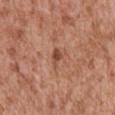Q: Is there a histopathology result?
A: no biopsy performed (imaged during a skin exam)
Q: How was this image acquired?
A: 15 mm crop, total-body photography
Q: How large is the lesion?
A: ~2.5 mm (longest diameter)
Q: How was the tile lit?
A: white-light
Q: Patient demographics?
A: male, aged 43 to 47
Q: What is the anatomic site?
A: the chest